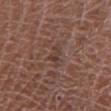Notes:
– biopsy status · total-body-photography surveillance lesion; no biopsy
– patient · male, aged around 75
– lighting · white-light illumination
– location · the left lower leg
– size · ≈3 mm
– imaging modality · ~15 mm tile from a whole-body skin photo
– automated metrics · a lesion area of about 3.5 mm² and an outline eccentricity of about 0.8 (0 = round, 1 = elongated); border irregularity of about 5 on a 0–10 scale, internal color variation of about 1 on a 0–10 scale, and radial color variation of about 0.5; an automated nevus-likeness rating near 0 out of 100 and lesion-presence confidence of about 70/100